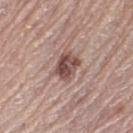{"biopsy_status": "not biopsied; imaged during a skin examination", "image": {"source": "total-body photography crop", "field_of_view_mm": 15}, "lighting": "white-light", "site": "right lower leg", "patient": {"sex": "female", "age_approx": 65}, "lesion_size": {"long_diameter_mm_approx": 3.5}}